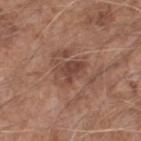<lesion>
  <biopsy_status>not biopsied; imaged during a skin examination</biopsy_status>
  <image>
    <source>total-body photography crop</source>
    <field_of_view_mm>15</field_of_view_mm>
  </image>
  <lesion_size>
    <long_diameter_mm_approx>3.0</long_diameter_mm_approx>
  </lesion_size>
  <lighting>white-light</lighting>
  <site>right forearm</site>
  <patient>
    <sex>male</sex>
    <age_approx>60</age_approx>
  </patient>
  <automated_metrics>
    <area_mm2_approx>5.0</area_mm2_approx>
    <eccentricity>0.65</eccentricity>
    <shape_asymmetry>0.4</shape_asymmetry>
    <cielab_L>43</cielab_L>
    <cielab_a>21</cielab_a>
    <cielab_b>25</cielab_b>
    <vs_skin_darker_L>9.0</vs_skin_darker_L>
    <vs_skin_contrast_norm>7.5</vs_skin_contrast_norm>
    <nevus_likeness_0_100>0</nevus_likeness_0_100>
  </automated_metrics>
</lesion>